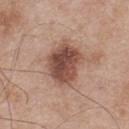{"site": "upper back", "automated_metrics": {"cielab_L": 48, "cielab_a": 21, "cielab_b": 26, "vs_skin_darker_L": 15.0, "vs_skin_contrast_norm": 10.5, "border_irregularity_0_10": 2.5}, "patient": {"sex": "male", "age_approx": 55}, "lesion_size": {"long_diameter_mm_approx": 5.0}, "image": {"source": "total-body photography crop", "field_of_view_mm": 15}}